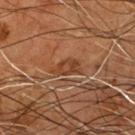Q: Was this lesion biopsied?
A: imaged on a skin check; not biopsied
Q: Where on the body is the lesion?
A: the chest
Q: What did automated image analysis measure?
A: an average lesion color of about L≈38 a*≈21 b*≈31 (CIELAB) and about 7 CIELAB-L* units darker than the surrounding skin; a border-irregularity rating of about 3/10 and a within-lesion color-variation index near 3.5/10
Q: How large is the lesion?
A: ~3.5 mm (longest diameter)
Q: Who is the patient?
A: male, aged approximately 55
Q: What kind of image is this?
A: ~15 mm crop, total-body skin-cancer survey